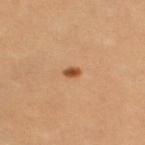<case>
  <biopsy_status>not biopsied; imaged during a skin examination</biopsy_status>
  <image>
    <source>total-body photography crop</source>
    <field_of_view_mm>15</field_of_view_mm>
  </image>
  <patient>
    <sex>female</sex>
    <age_approx>25</age_approx>
  </patient>
  <site>right thigh</site>
</case>